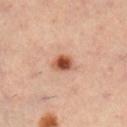Notes:
* follow-up — imaged on a skin check; not biopsied
* lesion size — about 3 mm
* imaging modality — ~15 mm tile from a whole-body skin photo
* anatomic site — the right lower leg
* patient — female, in their mid-30s